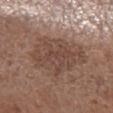biopsy status: imaged on a skin check; not biopsied
location: the left forearm
patient: male, about 55 years old
acquisition: ~15 mm crop, total-body skin-cancer survey
automated lesion analysis: a lesion area of about 24 mm², an eccentricity of roughly 0.65, and a shape-asymmetry score of about 0.3 (0 = symmetric); a lesion color around L≈45 a*≈17 b*≈24 in CIELAB, about 7 CIELAB-L* units darker than the surrounding skin, and a normalized lesion–skin contrast near 6
diameter: ~7 mm (longest diameter)
illumination: white-light